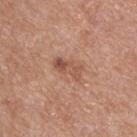<tbp_lesion>
<biopsy_status>not biopsied; imaged during a skin examination</biopsy_status>
<automated_metrics>
  <area_mm2_approx>5.0</area_mm2_approx>
  <eccentricity>0.9</eccentricity>
  <nevus_likeness_0_100>0</nevus_likeness_0_100>
  <lesion_detection_confidence_0_100>100</lesion_detection_confidence_0_100>
</automated_metrics>
<lighting>white-light</lighting>
<image>
  <source>total-body photography crop</source>
  <field_of_view_mm>15</field_of_view_mm>
</image>
<patient>
  <sex>male</sex>
  <age_approx>55</age_approx>
</patient>
<lesion_size>
  <long_diameter_mm_approx>3.5</long_diameter_mm_approx>
</lesion_size>
<site>upper back</site>
</tbp_lesion>